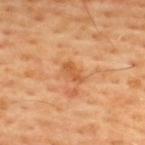Q: Is there a histopathology result?
A: imaged on a skin check; not biopsied
Q: How was the tile lit?
A: cross-polarized
Q: Automated lesion metrics?
A: a classifier nevus-likeness of about 0/100 and a detector confidence of about 100 out of 100 that the crop contains a lesion
Q: Lesion size?
A: about 2.5 mm
Q: Lesion location?
A: the upper back
Q: What is the imaging modality?
A: ~15 mm tile from a whole-body skin photo
Q: Patient demographics?
A: male, aged 53–57